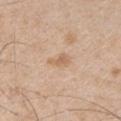Clinical impression: This lesion was catalogued during total-body skin photography and was not selected for biopsy. Acquisition and patient details: A male subject aged 48–52. A close-up tile cropped from a whole-body skin photograph, about 15 mm across. The lesion is located on the arm. About 3 mm across.